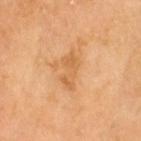Q: Is there a histopathology result?
A: catalogued during a skin exam; not biopsied
Q: What did automated image analysis measure?
A: a color-variation rating of about 1.5/10 and peripheral color asymmetry of about 0.5
Q: Patient demographics?
A: male, aged around 60
Q: How was this image acquired?
A: 15 mm crop, total-body photography
Q: What is the anatomic site?
A: the head or neck
Q: What lighting was used for the tile?
A: cross-polarized